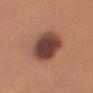biopsy_status: not biopsied; imaged during a skin examination
lighting: white-light
automated_metrics:
  area_mm2_approx: 18.0
  eccentricity: 0.6
  shape_asymmetry: 0.1
  peripheral_color_asymmetry: 1.5
lesion_size:
  long_diameter_mm_approx: 5.5
patient:
  sex: female
  age_approx: 35
site: left upper arm
image:
  source: total-body photography crop
  field_of_view_mm: 15